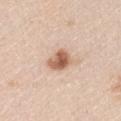Notes:
* notes: imaged on a skin check; not biopsied
* lesion diameter: ≈3 mm
* subject: male, aged 43 to 47
* automated lesion analysis: a lesion color around L≈61 a*≈20 b*≈31 in CIELAB, roughly 15 lightness units darker than nearby skin, and a lesion-to-skin contrast of about 9.5 (normalized; higher = more distinct); a classifier nevus-likeness of about 95/100 and a detector confidence of about 100 out of 100 that the crop contains a lesion
* image: 15 mm crop, total-body photography
* body site: the right upper arm